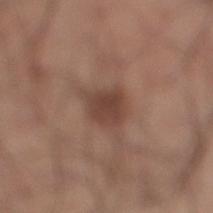Impression:
No biopsy was performed on this lesion — it was imaged during a full skin examination and was not determined to be concerning.
Context:
Automated tile analysis of the lesion measured a border-irregularity index near 3/10 and peripheral color asymmetry of about 1. A 15 mm crop from a total-body photograph taken for skin-cancer surveillance. A male patient, roughly 35 years of age. Imaged with white-light lighting. From the right lower leg.